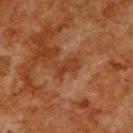- subject: male, aged 78 to 82
- TBP lesion metrics: a lesion color around L≈30 a*≈21 b*≈28 in CIELAB, roughly 6 lightness units darker than nearby skin, and a lesion-to-skin contrast of about 6.5 (normalized; higher = more distinct); internal color variation of about 1 on a 0–10 scale and radial color variation of about 0.5; a classifier nevus-likeness of about 0/100 and a lesion-detection confidence of about 100/100
- imaging modality: total-body-photography crop, ~15 mm field of view
- size: about 3 mm
- site: the upper back